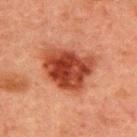Recorded during total-body skin imaging; not selected for excision or biopsy. The recorded lesion diameter is about 6 mm. A male patient, in their 50s. On the upper back. A 15 mm close-up tile from a total-body photography series done for melanoma screening. This is a cross-polarized tile. The lesion-visualizer software estimated a lesion area of about 23 mm², an outline eccentricity of about 0.65 (0 = round, 1 = elongated), and two-axis asymmetry of about 0.3.